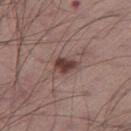notes: catalogued during a skin exam; not biopsied | illumination: white-light illumination | automated lesion analysis: a lesion color around L≈40 a*≈20 b*≈21 in CIELAB and a normalized border contrast of about 11; border irregularity of about 2.5 on a 0–10 scale, internal color variation of about 3 on a 0–10 scale, and peripheral color asymmetry of about 1; lesion-presence confidence of about 100/100 | subject: male, aged 53 to 57 | lesion diameter: about 2.5 mm | image source: ~15 mm tile from a whole-body skin photo | body site: the left thigh.